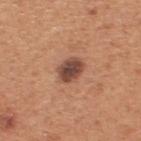The lesion was tiled from a total-body skin photograph and was not biopsied. This image is a 15 mm lesion crop taken from a total-body photograph. On the back. A male patient, aged around 55. Captured under white-light illumination. The lesion's longest dimension is about 3.5 mm.